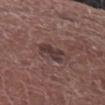follow-up = total-body-photography surveillance lesion; no biopsy | image source = ~15 mm tile from a whole-body skin photo | patient = male, roughly 70 years of age | lighting = white-light | automated metrics = a footprint of about 5.5 mm² and a symmetry-axis asymmetry near 0.4; a lesion color around L≈35 a*≈16 b*≈17 in CIELAB and about 9 CIELAB-L* units darker than the surrounding skin | diameter = about 3.5 mm | body site = the left forearm.